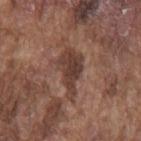Findings:
- follow-up — total-body-photography surveillance lesion; no biopsy
- body site — the mid back
- image — total-body-photography crop, ~15 mm field of view
- size — ≈5.5 mm
- patient — male, roughly 75 years of age